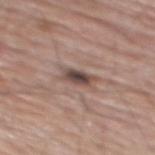follow-up = no biopsy performed (imaged during a skin exam) | subject = male, aged 78–82 | image = total-body-photography crop, ~15 mm field of view | lesion size = about 3.5 mm | body site = the mid back | automated metrics = an area of roughly 5 mm², an eccentricity of roughly 0.85, and two-axis asymmetry of about 0.25; a nevus-likeness score of about 0/100 and lesion-presence confidence of about 95/100.